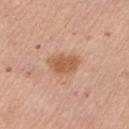Impression: The lesion was photographed on a routine skin check and not biopsied; there is no pathology result. Clinical summary: The lesion is located on the arm. The patient is a female in their mid- to late 60s. About 3.5 mm across. Captured under white-light illumination. A region of skin cropped from a whole-body photographic capture, roughly 15 mm wide.